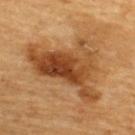workup: total-body-photography surveillance lesion; no biopsy | location: the back | size: about 10 mm | imaging modality: total-body-photography crop, ~15 mm field of view | subject: male, roughly 60 years of age | illumination: cross-polarized.